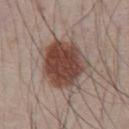The lesion is on the chest.
Longest diameter approximately 6.5 mm.
Cropped from a total-body skin-imaging series; the visible field is about 15 mm.
Imaged with white-light lighting.
A male subject, roughly 65 years of age.